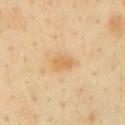Assessment: Imaged during a routine full-body skin examination; the lesion was not biopsied and no histopathology is available. Acquisition and patient details: A close-up tile cropped from a whole-body skin photograph, about 15 mm across. Located on the left upper arm. A male subject, in their 40s.